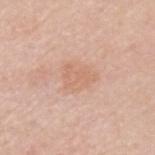Clinical impression:
The lesion was tiled from a total-body skin photograph and was not biopsied.
Acquisition and patient details:
The lesion is located on the upper back. The recorded lesion diameter is about 3.5 mm. A male subject, about 60 years old. An algorithmic analysis of the crop reported an average lesion color of about L≈66 a*≈20 b*≈31 (CIELAB), about 6 CIELAB-L* units darker than the surrounding skin, and a normalized lesion–skin contrast near 4.5. And it measured a border-irregularity rating of about 3/10 and internal color variation of about 1.5 on a 0–10 scale. A 15 mm crop from a total-body photograph taken for skin-cancer surveillance. This is a white-light tile.